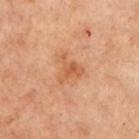This lesion was catalogued during total-body skin photography and was not selected for biopsy. The lesion's longest dimension is about 3.5 mm. Automated image analysis of the tile measured an area of roughly 6.5 mm² and a shape-asymmetry score of about 0.6 (0 = symmetric). It also reported a border-irregularity index near 6/10 and internal color variation of about 3 on a 0–10 scale. The lesion is on the chest. The subject is a female aged 53–57. Imaged with cross-polarized lighting. Cropped from a whole-body photographic skin survey; the tile spans about 15 mm.